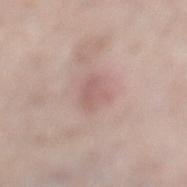biopsy_status: not biopsied; imaged during a skin examination
lighting: white-light
site: left lower leg
automated_metrics:
  area_mm2_approx: 6.0
  eccentricity: 0.7
  shape_asymmetry: 0.25
  border_irregularity_0_10: 2.5
  color_variation_0_10: 2.0
  peripheral_color_asymmetry: 0.5
patient:
  sex: female
  age_approx: 65
lesion_size:
  long_diameter_mm_approx: 3.0
image:
  source: total-body photography crop
  field_of_view_mm: 15